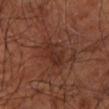Captured during whole-body skin photography for melanoma surveillance; the lesion was not biopsied.